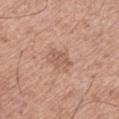subject: male, aged 63–67
imaging modality: ~15 mm crop, total-body skin-cancer survey
site: the left thigh